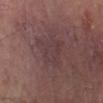Impression:
The lesion was photographed on a routine skin check and not biopsied; there is no pathology result.
Context:
From the left thigh. A region of skin cropped from a whole-body photographic capture, roughly 15 mm wide. A male patient, aged approximately 65.